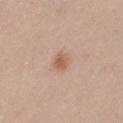The lesion was photographed on a routine skin check and not biopsied; there is no pathology result.
The patient is a female aged around 40.
Located on the chest.
Longest diameter approximately 2.5 mm.
Captured under white-light illumination.
A 15 mm close-up tile from a total-body photography series done for melanoma screening.
An algorithmic analysis of the crop reported an area of roughly 4 mm², a shape eccentricity near 0.55, and a symmetry-axis asymmetry near 0.2. And it measured lesion-presence confidence of about 100/100.